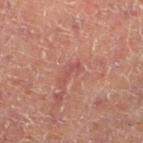The lesion was photographed on a routine skin check and not biopsied; there is no pathology result.
Located on the left lower leg.
The patient is a male aged around 50.
The recorded lesion diameter is about 2.5 mm.
A 15 mm crop from a total-body photograph taken for skin-cancer surveillance.
This is a cross-polarized tile.